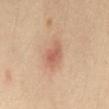workup: catalogued during a skin exam; not biopsied | body site: the abdomen | patient: female, in their 40s | diameter: ~4 mm (longest diameter) | image: ~15 mm crop, total-body skin-cancer survey | automated lesion analysis: a shape eccentricity near 0.85 and two-axis asymmetry of about 0.25; border irregularity of about 2.5 on a 0–10 scale, a color-variation rating of about 3/10, and peripheral color asymmetry of about 1 | illumination: cross-polarized illumination.